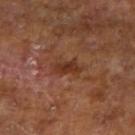Q: Was a biopsy performed?
A: total-body-photography surveillance lesion; no biopsy
Q: What is the imaging modality?
A: ~15 mm crop, total-body skin-cancer survey
Q: Where on the body is the lesion?
A: the arm
Q: Lesion size?
A: about 3.5 mm
Q: Who is the patient?
A: male, about 65 years old
Q: Illumination type?
A: cross-polarized